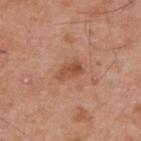Findings:
* workup · imaged on a skin check; not biopsied
* location · the upper back
* automated lesion analysis · a mean CIELAB color near L≈51 a*≈24 b*≈32, about 9 CIELAB-L* units darker than the surrounding skin, and a normalized lesion–skin contrast near 6.5; border irregularity of about 3 on a 0–10 scale, a within-lesion color-variation index near 3/10, and a peripheral color-asymmetry measure near 1
* lighting · white-light illumination
* diameter · about 3.5 mm
* subject · male, in their mid-50s
* image · total-body-photography crop, ~15 mm field of view